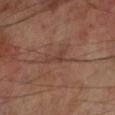A 15 mm close-up tile from a total-body photography series done for melanoma screening. Captured under cross-polarized illumination. Longest diameter approximately 3 mm. The lesion-visualizer software estimated a lesion area of about 2.5 mm² and a shape eccentricity near 0.85. And it measured a color-variation rating of about 0/10. The software also gave a classifier nevus-likeness of about 0/100. A male patient about 70 years old. From the left lower leg.